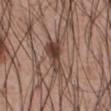Clinical impression: No biopsy was performed on this lesion — it was imaged during a full skin examination and was not determined to be concerning.